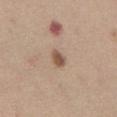Assessment: No biopsy was performed on this lesion — it was imaged during a full skin examination and was not determined to be concerning. Image and clinical context: Measured at roughly 2.5 mm in maximum diameter. Located on the abdomen. A close-up tile cropped from a whole-body skin photograph, about 15 mm across. The patient is a male about 60 years old. Automated image analysis of the tile measured about 12 CIELAB-L* units darker than the surrounding skin and a normalized border contrast of about 8.5. The analysis additionally found a border-irregularity rating of about 1.5/10, a color-variation rating of about 2/10, and peripheral color asymmetry of about 0.5.